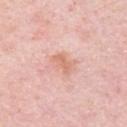biopsy_status: not biopsied; imaged during a skin examination
lighting: white-light
patient:
  sex: female
  age_approx: 65
site: left upper arm
image:
  source: total-body photography crop
  field_of_view_mm: 15
lesion_size:
  long_diameter_mm_approx: 2.5